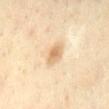Measured at roughly 2.5 mm in maximum diameter.
A 15 mm close-up tile from a total-body photography series done for melanoma screening.
The lesion is located on the mid back.
A female patient roughly 60 years of age.
Imaged with cross-polarized lighting.